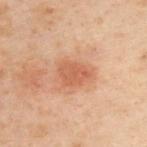  biopsy_status: not biopsied; imaged during a skin examination
  image:
    source: total-body photography crop
    field_of_view_mm: 15
  lighting: cross-polarized
  site: upper back
  patient:
    sex: male
    age_approx: 50
  lesion_size:
    long_diameter_mm_approx: 3.5